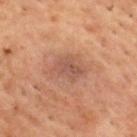Recorded during total-body skin imaging; not selected for excision or biopsy.
The total-body-photography lesion software estimated a lesion area of about 10 mm² and an outline eccentricity of about 0.7 (0 = round, 1 = elongated). It also reported a border-irregularity rating of about 2.5/10 and a peripheral color-asymmetry measure near 1. It also reported a detector confidence of about 100 out of 100 that the crop contains a lesion.
The lesion is located on the upper back.
This image is a 15 mm lesion crop taken from a total-body photograph.
The patient is a male aged 53 to 57.
The lesion's longest dimension is about 4 mm.
The tile uses cross-polarized illumination.